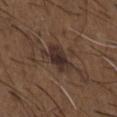No biopsy was performed on this lesion — it was imaged during a full skin examination and was not determined to be concerning. From the chest. A male patient roughly 50 years of age. An algorithmic analysis of the crop reported a lesion area of about 8.5 mm² and a shape eccentricity near 0.8. And it measured a within-lesion color-variation index near 3.5/10 and peripheral color asymmetry of about 1. Captured under white-light illumination. Longest diameter approximately 4.5 mm. A 15 mm crop from a total-body photograph taken for skin-cancer surveillance.